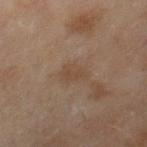workup = no biopsy performed (imaged during a skin exam)
lighting = cross-polarized
subject = female, aged around 60
acquisition = total-body-photography crop, ~15 mm field of view
diameter = about 3 mm
body site = the right thigh